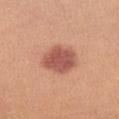Imaged during a routine full-body skin examination; the lesion was not biopsied and no histopathology is available.